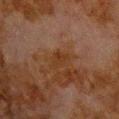| feature | finding |
|---|---|
| automated metrics | an average lesion color of about L≈27 a*≈17 b*≈27 (CIELAB), a lesion–skin lightness drop of about 4, and a normalized lesion–skin contrast near 6; an automated nevus-likeness rating near 0 out of 100 and a lesion-detection confidence of about 100/100 |
| imaging modality | 15 mm crop, total-body photography |
| subject | male, in their 80s |
| lighting | cross-polarized illumination |
| size | ~2.5 mm (longest diameter) |
| anatomic site | the upper back |